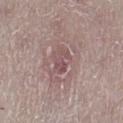Impression: Recorded during total-body skin imaging; not selected for excision or biopsy. Background: The lesion's longest dimension is about 3 mm. A 15 mm close-up tile from a total-body photography series done for melanoma screening. The patient is a female aged 38 to 42. From the right lower leg.